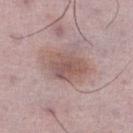Case summary:
- lesion diameter — ~5 mm (longest diameter)
- location — the right lower leg
- subject — male, aged approximately 50
- imaging modality — ~15 mm crop, total-body skin-cancer survey
- automated metrics — a lesion area of about 13 mm² and a shape-asymmetry score of about 0.2 (0 = symmetric); internal color variation of about 4 on a 0–10 scale and radial color variation of about 1.5; a nevus-likeness score of about 20/100 and a detector confidence of about 100 out of 100 that the crop contains a lesion